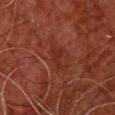Part of a total-body skin-imaging series; this lesion was reviewed on a skin check and was not flagged for biopsy. The lesion-visualizer software estimated an eccentricity of roughly 0.9 and a symmetry-axis asymmetry near 0.5. This image is a 15 mm lesion crop taken from a total-body photograph. The lesion is on the chest. The subject is a male approximately 60 years of age. The recorded lesion diameter is about 3.5 mm.